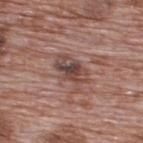subject: male, aged around 70 | anatomic site: the upper back | acquisition: ~15 mm tile from a whole-body skin photo | tile lighting: white-light | automated lesion analysis: a footprint of about 9.5 mm² and a symmetry-axis asymmetry near 0.3; a mean CIELAB color near L≈45 a*≈19 b*≈22, about 11 CIELAB-L* units darker than the surrounding skin, and a lesion-to-skin contrast of about 8 (normalized; higher = more distinct); a border-irregularity index near 4.5/10 and a peripheral color-asymmetry measure near 2.5; a classifier nevus-likeness of about 0/100 and lesion-presence confidence of about 85/100.